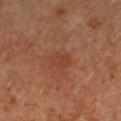biopsy status: catalogued during a skin exam; not biopsied | subject: female, roughly 50 years of age | size: ≈3 mm | site: the right forearm | lighting: cross-polarized illumination | acquisition: ~15 mm tile from a whole-body skin photo.